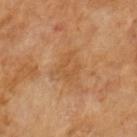acquisition: total-body-photography crop, ~15 mm field of view
patient: male, in their mid-60s
tile lighting: cross-polarized illumination